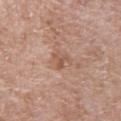Impression: The lesion was photographed on a routine skin check and not biopsied; there is no pathology result. Clinical summary: The lesion-visualizer software estimated a footprint of about 3.5 mm², an outline eccentricity of about 0.7 (0 = round, 1 = elongated), and a shape-asymmetry score of about 0.5 (0 = symmetric). And it measured a lesion color around L≈54 a*≈20 b*≈29 in CIELAB, about 8 CIELAB-L* units darker than the surrounding skin, and a normalized lesion–skin contrast near 6. On the left upper arm. A female patient approximately 70 years of age. Cropped from a total-body skin-imaging series; the visible field is about 15 mm. Longest diameter approximately 3 mm. This is a white-light tile.